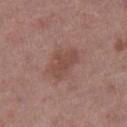Notes:
– biopsy status — no biopsy performed (imaged during a skin exam)
– lesion size — ≈4.5 mm
– subject — male, about 70 years old
– illumination — white-light
– image source — 15 mm crop, total-body photography
– body site — the right thigh